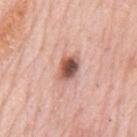Q: Was this lesion biopsied?
A: no biopsy performed (imaged during a skin exam)
Q: What is the imaging modality?
A: ~15 mm crop, total-body skin-cancer survey
Q: Automated lesion metrics?
A: a shape eccentricity near 0.7 and a symmetry-axis asymmetry near 0.2; a mean CIELAB color near L≈54 a*≈23 b*≈26, about 18 CIELAB-L* units darker than the surrounding skin, and a lesion-to-skin contrast of about 11.5 (normalized; higher = more distinct)
Q: What is the lesion's diameter?
A: ≈3 mm
Q: Illumination type?
A: white-light illumination
Q: What is the anatomic site?
A: the mid back
Q: What are the patient's age and sex?
A: male, roughly 80 years of age